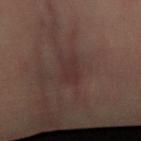<case>
<biopsy_status>not biopsied; imaged during a skin examination</biopsy_status>
<site>left forearm</site>
<automated_metrics>
  <eccentricity>0.8</eccentricity>
  <shape_asymmetry>0.2</shape_asymmetry>
  <border_irregularity_0_10>2.0</border_irregularity_0_10>
  <color_variation_0_10>2.0</color_variation_0_10>
</automated_metrics>
<lesion_size>
  <long_diameter_mm_approx>3.5</long_diameter_mm_approx>
</lesion_size>
<image>
  <source>total-body photography crop</source>
  <field_of_view_mm>15</field_of_view_mm>
</image>
<lighting>cross-polarized</lighting>
<patient>
  <sex>male</sex>
  <age_approx>50</age_approx>
</patient>
</case>